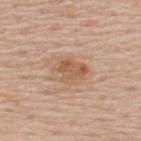<tbp_lesion>
<lesion_size>
  <long_diameter_mm_approx>4.0</long_diameter_mm_approx>
</lesion_size>
<lighting>white-light</lighting>
<site>upper back</site>
<patient>
  <sex>male</sex>
  <age_approx>60</age_approx>
</patient>
<image>
  <source>total-body photography crop</source>
  <field_of_view_mm>15</field_of_view_mm>
</image>
</tbp_lesion>